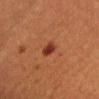Recorded during total-body skin imaging; not selected for excision or biopsy. The total-body-photography lesion software estimated a lesion area of about 3 mm² and an outline eccentricity of about 0.85 (0 = round, 1 = elongated). Located on the head or neck. Cropped from a total-body skin-imaging series; the visible field is about 15 mm. A male subject roughly 40 years of age.